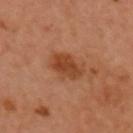Imaged during a routine full-body skin examination; the lesion was not biopsied and no histopathology is available. The lesion is located on the head or neck. A close-up tile cropped from a whole-body skin photograph, about 15 mm across. The subject is a female aged around 60. Captured under cross-polarized illumination. The lesion's longest dimension is about 4 mm.